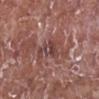The lesion was tiled from a total-body skin photograph and was not biopsied. A male subject, roughly 75 years of age. A 15 mm close-up extracted from a 3D total-body photography capture. The total-body-photography lesion software estimated a lesion area of about 6.5 mm², a shape eccentricity near 0.8, and a shape-asymmetry score of about 0.55 (0 = symmetric). The analysis additionally found an average lesion color of about L≈43 a*≈21 b*≈21 (CIELAB), roughly 8 lightness units darker than nearby skin, and a normalized border contrast of about 7. The analysis additionally found a classifier nevus-likeness of about 0/100 and lesion-presence confidence of about 60/100. The tile uses white-light illumination. Approximately 4 mm at its widest. The lesion is located on the right lower leg.